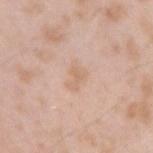notes: no biopsy performed (imaged during a skin exam) | diameter: ~3 mm (longest diameter) | tile lighting: white-light | patient: male, in their mid- to late 20s | location: the right upper arm | image: ~15 mm crop, total-body skin-cancer survey | TBP lesion metrics: an area of roughly 4.5 mm² and an eccentricity of roughly 0.8; a lesion color around L≈67 a*≈18 b*≈30 in CIELAB, a lesion–skin lightness drop of about 6, and a lesion-to-skin contrast of about 4.5 (normalized; higher = more distinct).